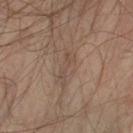Recorded during total-body skin imaging; not selected for excision or biopsy. From the left lower leg. A male subject, aged 43 to 47. Approximately 4 mm at its widest. A lesion tile, about 15 mm wide, cut from a 3D total-body photograph. An algorithmic analysis of the crop reported two-axis asymmetry of about 0.4. And it measured an average lesion color of about L≈46 a*≈15 b*≈24 (CIELAB), a lesion–skin lightness drop of about 6, and a normalized border contrast of about 5. The analysis additionally found border irregularity of about 5.5 on a 0–10 scale, a color-variation rating of about 1.5/10, and a peripheral color-asymmetry measure near 0.5. This is a cross-polarized tile.